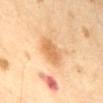biopsy status — no biopsy performed (imaged during a skin exam); imaging modality — ~15 mm crop, total-body skin-cancer survey; tile lighting — cross-polarized illumination; subject — male, approximately 65 years of age; lesion size — about 4 mm.